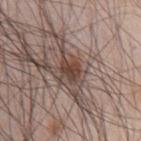Clinical impression:
The lesion was photographed on a routine skin check and not biopsied; there is no pathology result.
Context:
A male subject, roughly 60 years of age. The lesion is located on the chest. A 15 mm crop from a total-body photograph taken for skin-cancer surveillance. The lesion's longest dimension is about 2.5 mm. This is a white-light tile.